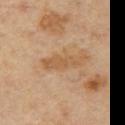biopsy status: total-body-photography surveillance lesion; no biopsy
TBP lesion metrics: a lesion area of about 6.5 mm² and an outline eccentricity of about 0.95 (0 = round, 1 = elongated)
body site: the left upper arm
lighting: cross-polarized illumination
subject: male, aged approximately 55
image source: 15 mm crop, total-body photography
lesion diameter: ≈5 mm